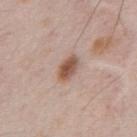Clinical impression: No biopsy was performed on this lesion — it was imaged during a full skin examination and was not determined to be concerning. Acquisition and patient details: A male subject, aged 48–52. The tile uses white-light illumination. From the chest. The total-body-photography lesion software estimated an area of roughly 5.5 mm², an outline eccentricity of about 0.8 (0 = round, 1 = elongated), and a symmetry-axis asymmetry near 0.2. The software also gave internal color variation of about 3.5 on a 0–10 scale and radial color variation of about 1. A lesion tile, about 15 mm wide, cut from a 3D total-body photograph.